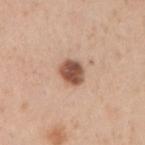Q: Was this lesion biopsied?
A: no biopsy performed (imaged during a skin exam)